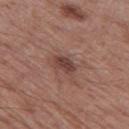<lesion>
  <biopsy_status>not biopsied; imaged during a skin examination</biopsy_status>
  <site>left thigh</site>
  <image>
    <source>total-body photography crop</source>
    <field_of_view_mm>15</field_of_view_mm>
  </image>
  <patient>
    <sex>male</sex>
    <age_approx>55</age_approx>
  </patient>
</lesion>